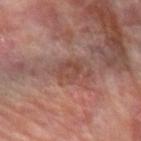{
  "biopsy_status": "not biopsied; imaged during a skin examination",
  "patient": {
    "sex": "female",
    "age_approx": 65
  },
  "site": "left forearm",
  "lesion_size": {
    "long_diameter_mm_approx": 4.0
  },
  "image": {
    "source": "total-body photography crop",
    "field_of_view_mm": 15
  },
  "lighting": "cross-polarized"
}